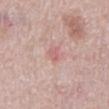Part of a total-body skin-imaging series; this lesion was reviewed on a skin check and was not flagged for biopsy.
A male patient, roughly 65 years of age.
Located on the mid back.
A close-up tile cropped from a whole-body skin photograph, about 15 mm across.
The recorded lesion diameter is about 2.5 mm.
The total-body-photography lesion software estimated an outline eccentricity of about 0.85 (0 = round, 1 = elongated) and a symmetry-axis asymmetry near 0.3. It also reported a mean CIELAB color near L≈62 a*≈25 b*≈22, roughly 7 lightness units darker than nearby skin, and a normalized lesion–skin contrast near 4.5.
Imaged with white-light lighting.